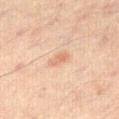Imaged during a routine full-body skin examination; the lesion was not biopsied and no histopathology is available.
A 15 mm close-up extracted from a 3D total-body photography capture.
Measured at roughly 2.5 mm in maximum diameter.
Located on the leg.
Captured under cross-polarized illumination.
A male patient, in their 60s.